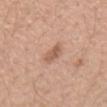{"biopsy_status": "not biopsied; imaged during a skin examination", "site": "right upper arm", "automated_metrics": {"area_mm2_approx": 4.5, "eccentricity": 0.75, "shape_asymmetry": 0.4, "cielab_L": 58, "cielab_a": 21, "cielab_b": 30, "vs_skin_darker_L": 10.0, "vs_skin_contrast_norm": 6.5, "nevus_likeness_0_100": 50, "lesion_detection_confidence_0_100": 100}, "lesion_size": {"long_diameter_mm_approx": 3.0}, "patient": {"sex": "female", "age_approx": 45}, "image": {"source": "total-body photography crop", "field_of_view_mm": 15}, "lighting": "white-light"}